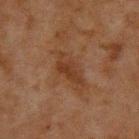Impression:
The lesion was photographed on a routine skin check and not biopsied; there is no pathology result.
Image and clinical context:
A male patient about 60 years old. About 4 mm across. Captured under cross-polarized illumination. A region of skin cropped from a whole-body photographic capture, roughly 15 mm wide. On the upper back.